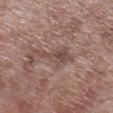workup — imaged on a skin check; not biopsied
patient — male, in their mid-50s
body site — the right lower leg
imaging modality — total-body-photography crop, ~15 mm field of view
lesion diameter — ~5 mm (longest diameter)